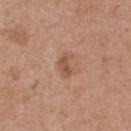Part of a total-body skin-imaging series; this lesion was reviewed on a skin check and was not flagged for biopsy.
A roughly 15 mm field-of-view crop from a total-body skin photograph.
Located on the back.
The patient is a female aged approximately 40.